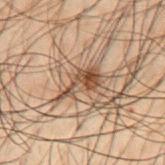Q: Patient demographics?
A: male, roughly 50 years of age
Q: What is the anatomic site?
A: the back
Q: What kind of image is this?
A: total-body-photography crop, ~15 mm field of view